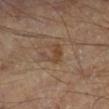notes — catalogued during a skin exam; not biopsied
automated lesion analysis — a shape eccentricity near 0.5; a lesion color around L≈42 a*≈16 b*≈28 in CIELAB, a lesion–skin lightness drop of about 7, and a lesion-to-skin contrast of about 6 (normalized; higher = more distinct); internal color variation of about 1.5 on a 0–10 scale and a peripheral color-asymmetry measure near 0.5; a nevus-likeness score of about 0/100 and a detector confidence of about 100 out of 100 that the crop contains a lesion
anatomic site — the right lower leg
lesion diameter — ≈3 mm
lighting — cross-polarized
patient — male, aged around 70
image — ~15 mm crop, total-body skin-cancer survey